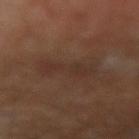The lesion was photographed on a routine skin check and not biopsied; there is no pathology result. Located on the right upper arm. A male patient about 55 years old. Captured under cross-polarized illumination. A close-up tile cropped from a whole-body skin photograph, about 15 mm across. The lesion's longest dimension is about 5 mm.